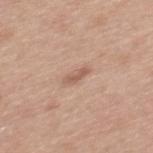* workup: no biopsy performed (imaged during a skin exam)
* anatomic site: the mid back
* subject: male, aged around 30
* tile lighting: white-light
* image-analysis metrics: an outline eccentricity of about 0.9 (0 = round, 1 = elongated) and two-axis asymmetry of about 0.3; a lesion color around L≈57 a*≈20 b*≈29 in CIELAB, about 9 CIELAB-L* units darker than the surrounding skin, and a normalized border contrast of about 6.5
* image source: ~15 mm tile from a whole-body skin photo